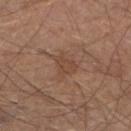Assessment:
Part of a total-body skin-imaging series; this lesion was reviewed on a skin check and was not flagged for biopsy.
Background:
From the left lower leg. The tile uses white-light illumination. A 15 mm close-up tile from a total-body photography series done for melanoma screening. A male subject, approximately 65 years of age. Approximately 3 mm at its widest.